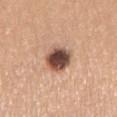Impression: Recorded during total-body skin imaging; not selected for excision or biopsy. Clinical summary: The recorded lesion diameter is about 3.5 mm. Imaged with white-light lighting. The subject is a female aged 63–67. Automated tile analysis of the lesion measured an area of roughly 10 mm² and an eccentricity of roughly 0.55. The software also gave a mean CIELAB color near L≈49 a*≈20 b*≈27, roughly 20 lightness units darker than nearby skin, and a normalized border contrast of about 13.5. The software also gave internal color variation of about 7.5 on a 0–10 scale and peripheral color asymmetry of about 1.5. A 15 mm close-up extracted from a 3D total-body photography capture. The lesion is located on the right upper arm.